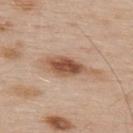<record>
  <biopsy_status>not biopsied; imaged during a skin examination</biopsy_status>
  <site>upper back</site>
  <lesion_size>
    <long_diameter_mm_approx>6.5</long_diameter_mm_approx>
  </lesion_size>
  <patient>
    <sex>male</sex>
    <age_approx>55</age_approx>
  </patient>
  <lighting>white-light</lighting>
  <image>
    <source>total-body photography crop</source>
    <field_of_view_mm>15</field_of_view_mm>
  </image>
</record>